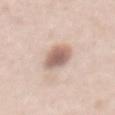Q: Was a biopsy performed?
A: no biopsy performed (imaged during a skin exam)
Q: What did automated image analysis measure?
A: a footprint of about 9 mm² and an eccentricity of roughly 0.85; internal color variation of about 4.5 on a 0–10 scale and peripheral color asymmetry of about 1.5; a classifier nevus-likeness of about 100/100
Q: How was the tile lit?
A: white-light
Q: Where on the body is the lesion?
A: the front of the torso
Q: What is the imaging modality?
A: ~15 mm crop, total-body skin-cancer survey
Q: Patient demographics?
A: male, roughly 50 years of age
Q: How large is the lesion?
A: ~4.5 mm (longest diameter)